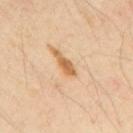Notes:
– biopsy status: total-body-photography surveillance lesion; no biopsy
– imaging modality: 15 mm crop, total-body photography
– site: the back
– diameter: ≈3 mm
– lighting: cross-polarized
– patient: male, about 50 years old